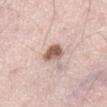Q: Was this lesion biopsied?
A: no biopsy performed (imaged during a skin exam)
Q: What lighting was used for the tile?
A: white-light illumination
Q: What kind of image is this?
A: ~15 mm tile from a whole-body skin photo
Q: Lesion size?
A: ≈3 mm
Q: What are the patient's age and sex?
A: male, aged 68–72
Q: What did automated image analysis measure?
A: an area of roughly 5.5 mm², a shape eccentricity near 0.6, and a symmetry-axis asymmetry near 0.3; an automated nevus-likeness rating near 90 out of 100 and lesion-presence confidence of about 100/100
Q: Lesion location?
A: the abdomen